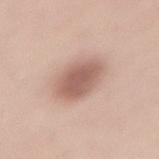Captured during whole-body skin photography for melanoma surveillance; the lesion was not biopsied.
A male subject, roughly 55 years of age.
On the lower back.
The lesion's longest dimension is about 4.5 mm.
The tile uses white-light illumination.
Cropped from a total-body skin-imaging series; the visible field is about 15 mm.